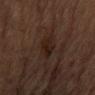Part of a total-body skin-imaging series; this lesion was reviewed on a skin check and was not flagged for biopsy. Imaged with cross-polarized lighting. A male subject, aged 83 or over. The lesion is located on the lower back. Longest diameter approximately 4.5 mm. A roughly 15 mm field-of-view crop from a total-body skin photograph.